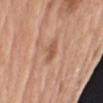Imaged during a routine full-body skin examination; the lesion was not biopsied and no histopathology is available. On the right upper arm. The total-body-photography lesion software estimated internal color variation of about 1.5 on a 0–10 scale and peripheral color asymmetry of about 0.5. And it measured an automated nevus-likeness rating near 0 out of 100 and a lesion-detection confidence of about 100/100. A female patient, approximately 75 years of age. The tile uses white-light illumination. The lesion's longest dimension is about 2.5 mm. A 15 mm close-up extracted from a 3D total-body photography capture.